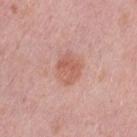biopsy status = total-body-photography surveillance lesion; no biopsy | body site = the left thigh | lighting = white-light illumination | lesion diameter = ~3 mm (longest diameter) | image source = 15 mm crop, total-body photography | patient = female, roughly 40 years of age.